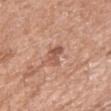Impression:
The lesion was tiled from a total-body skin photograph and was not biopsied.
Context:
Automated image analysis of the tile measured an average lesion color of about L≈54 a*≈24 b*≈29 (CIELAB) and a normalized lesion–skin contrast near 7. It also reported an automated nevus-likeness rating near 0 out of 100 and lesion-presence confidence of about 100/100. Imaged with white-light lighting. Cropped from a total-body skin-imaging series; the visible field is about 15 mm. Approximately 3 mm at its widest. The lesion is on the right upper arm. A female subject aged 73 to 77.